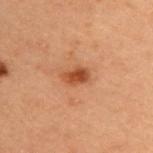Assessment:
No biopsy was performed on this lesion — it was imaged during a full skin examination and was not determined to be concerning.
Context:
From the upper back. A female subject, aged around 40. A close-up tile cropped from a whole-body skin photograph, about 15 mm across.